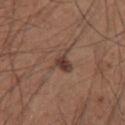Imaged during a routine full-body skin examination; the lesion was not biopsied and no histopathology is available.
Automated tile analysis of the lesion measured a lesion area of about 5.5 mm² and an eccentricity of roughly 0.65. It also reported a border-irregularity index near 5.5/10, a color-variation rating of about 3/10, and radial color variation of about 1.
About 3.5 mm across.
Imaged with white-light lighting.
Located on the chest.
A 15 mm crop from a total-body photograph taken for skin-cancer surveillance.
A male subject aged around 60.